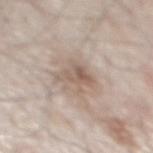Assessment:
This lesion was catalogued during total-body skin photography and was not selected for biopsy.
Acquisition and patient details:
The tile uses white-light illumination. About 3.5 mm across. A 15 mm crop from a total-body photograph taken for skin-cancer surveillance. A male subject, aged approximately 80. The total-body-photography lesion software estimated a footprint of about 7.5 mm² and a shape eccentricity near 0.55. The analysis additionally found a mean CIELAB color near L≈58 a*≈14 b*≈25 and a normalized border contrast of about 6.5. It also reported border irregularity of about 5 on a 0–10 scale and internal color variation of about 5.5 on a 0–10 scale. The lesion is on the mid back.